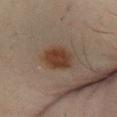• biopsy status — total-body-photography surveillance lesion; no biopsy
• lesion diameter — ~4 mm (longest diameter)
• image source — ~15 mm crop, total-body skin-cancer survey
• anatomic site — the right lower leg
• patient — male, approximately 65 years of age
• tile lighting — cross-polarized illumination